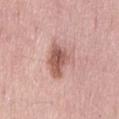biopsy_status: not biopsied; imaged during a skin examination
site: lower back
image:
  source: total-body photography crop
  field_of_view_mm: 15
lesion_size:
  long_diameter_mm_approx: 5.0
lighting: white-light
automated_metrics:
  eccentricity: 0.8
  shape_asymmetry: 0.4
patient:
  sex: female
  age_approx: 50